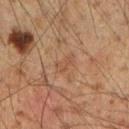Recorded during total-body skin imaging; not selected for excision or biopsy.
A male patient, aged 48 to 52.
An algorithmic analysis of the crop reported a within-lesion color-variation index near 0/10 and radial color variation of about 0. The analysis additionally found a nevus-likeness score of about 0/100 and lesion-presence confidence of about 100/100.
The lesion is on the right upper arm.
This is a cross-polarized tile.
A 15 mm close-up extracted from a 3D total-body photography capture.
Longest diameter approximately 3 mm.